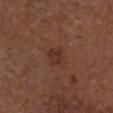No biopsy was performed on this lesion — it was imaged during a full skin examination and was not determined to be concerning. The lesion-visualizer software estimated an average lesion color of about L≈32 a*≈20 b*≈25 (CIELAB) and a lesion-to-skin contrast of about 6.5 (normalized; higher = more distinct). It also reported a border-irregularity rating of about 2/10, a within-lesion color-variation index near 3/10, and a peripheral color-asymmetry measure near 1. It also reported a detector confidence of about 100 out of 100 that the crop contains a lesion. Longest diameter approximately 3 mm. This image is a 15 mm lesion crop taken from a total-body photograph. Located on the head or neck. The tile uses white-light illumination. The patient is a male roughly 75 years of age.